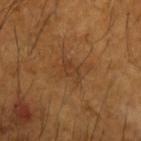imaging modality = total-body-photography crop, ~15 mm field of view; patient = male, approximately 65 years of age; location = the left forearm; illumination = cross-polarized.